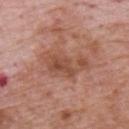{"biopsy_status": "not biopsied; imaged during a skin examination", "patient": {"sex": "male", "age_approx": 70}, "image": {"source": "total-body photography crop", "field_of_view_mm": 15}, "site": "upper back", "lighting": "white-light", "lesion_size": {"long_diameter_mm_approx": 6.5}}